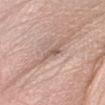follow-up: total-body-photography surveillance lesion; no biopsy
subject: female, aged 33 to 37
anatomic site: the arm
imaging modality: 15 mm crop, total-body photography
tile lighting: white-light
diameter: about 3 mm